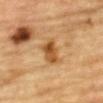Clinical summary:
The lesion is on the chest. Automated tile analysis of the lesion measured a lesion area of about 7.5 mm² and a shape-asymmetry score of about 0.3 (0 = symmetric). The analysis additionally found a border-irregularity index near 3/10 and a color-variation rating of about 4.5/10. The analysis additionally found a classifier nevus-likeness of about 55/100 and a detector confidence of about 100 out of 100 that the crop contains a lesion. A region of skin cropped from a whole-body photographic capture, roughly 15 mm wide. A male patient aged approximately 85 or older. The recorded lesion diameter is about 3.5 mm. Imaged with cross-polarized lighting.